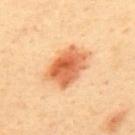follow-up: catalogued during a skin exam; not biopsied
subject: male, approximately 40 years of age
lesion diameter: ~5 mm (longest diameter)
imaging modality: ~15 mm tile from a whole-body skin photo
image-analysis metrics: a border-irregularity rating of about 2/10 and a peripheral color-asymmetry measure near 2.5; an automated nevus-likeness rating near 100 out of 100 and a detector confidence of about 100 out of 100 that the crop contains a lesion
location: the mid back